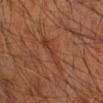notes — total-body-photography surveillance lesion; no biopsy
location — the right forearm
image source — ~15 mm tile from a whole-body skin photo
lighting — cross-polarized illumination
image-analysis metrics — a footprint of about 5.5 mm², a shape eccentricity near 0.95, and two-axis asymmetry of about 0.4; about 6 CIELAB-L* units darker than the surrounding skin; internal color variation of about 2 on a 0–10 scale and radial color variation of about 0.5; an automated nevus-likeness rating near 0 out of 100 and a detector confidence of about 70 out of 100 that the crop contains a lesion
patient — male, aged 58 to 62
size — ≈4.5 mm